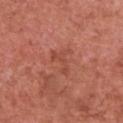<record>
  <biopsy_status>not biopsied; imaged during a skin examination</biopsy_status>
  <lighting>white-light</lighting>
  <patient>
    <sex>female</sex>
    <age_approx>50</age_approx>
  </patient>
  <lesion_size>
    <long_diameter_mm_approx>3.5</long_diameter_mm_approx>
  </lesion_size>
  <site>chest</site>
  <image>
    <source>total-body photography crop</source>
    <field_of_view_mm>15</field_of_view_mm>
  </image>
</record>